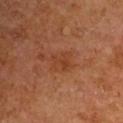follow-up: imaged on a skin check; not biopsied
image-analysis metrics: a shape eccentricity near 0.4 and a shape-asymmetry score of about 0.35 (0 = symmetric); border irregularity of about 4 on a 0–10 scale and a within-lesion color-variation index near 2/10; an automated nevus-likeness rating near 0 out of 100 and a lesion-detection confidence of about 100/100
lesion size: ~3 mm (longest diameter)
patient: male, roughly 65 years of age
tile lighting: cross-polarized illumination
image: ~15 mm crop, total-body skin-cancer survey
anatomic site: the left upper arm